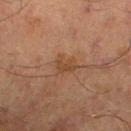Impression:
Part of a total-body skin-imaging series; this lesion was reviewed on a skin check and was not flagged for biopsy.
Clinical summary:
The lesion is located on the right lower leg. Longest diameter approximately 3 mm. A roughly 15 mm field-of-view crop from a total-body skin photograph. Imaged with cross-polarized lighting. A male patient aged around 70.